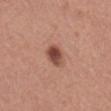Imaged during a routine full-body skin examination; the lesion was not biopsied and no histopathology is available.
Located on the mid back.
A 15 mm crop from a total-body photograph taken for skin-cancer surveillance.
A male subject in their mid-50s.